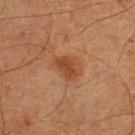Recorded during total-body skin imaging; not selected for excision or biopsy. The recorded lesion diameter is about 3.5 mm. A 15 mm close-up extracted from a 3D total-body photography capture. This is a cross-polarized tile. A male patient approximately 65 years of age. From the right lower leg.